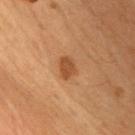Q: Was a biopsy performed?
A: total-body-photography surveillance lesion; no biopsy
Q: Lesion location?
A: the chest
Q: What kind of image is this?
A: 15 mm crop, total-body photography
Q: How was the tile lit?
A: cross-polarized
Q: What did automated image analysis measure?
A: a nevus-likeness score of about 90/100 and a lesion-detection confidence of about 100/100
Q: Patient demographics?
A: male, aged 48–52